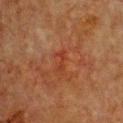Clinical impression:
Captured during whole-body skin photography for melanoma surveillance; the lesion was not biopsied.
Acquisition and patient details:
Captured under cross-polarized illumination. The lesion is on the chest. A close-up tile cropped from a whole-body skin photograph, about 15 mm across. The recorded lesion diameter is about 2.5 mm. A female subject, about 55 years old. The total-body-photography lesion software estimated a lesion area of about 2.5 mm², an outline eccentricity of about 0.95 (0 = round, 1 = elongated), and a symmetry-axis asymmetry near 0.35. The software also gave an automated nevus-likeness rating near 0 out of 100 and a lesion-detection confidence of about 100/100.